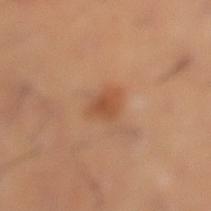The lesion was photographed on a routine skin check and not biopsied; there is no pathology result. The lesion-visualizer software estimated a classifier nevus-likeness of about 80/100 and a detector confidence of about 100 out of 100 that the crop contains a lesion. A male subject, approximately 30 years of age. Longest diameter approximately 3 mm. Located on the left lower leg. Captured under cross-polarized illumination. A close-up tile cropped from a whole-body skin photograph, about 15 mm across.